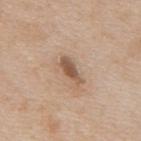Notes:
– notes: no biopsy performed (imaged during a skin exam)